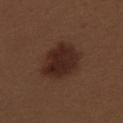Recorded during total-body skin imaging; not selected for excision or biopsy.
The lesion is on the upper back.
A female subject about 30 years old.
Cropped from a total-body skin-imaging series; the visible field is about 15 mm.